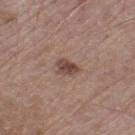No biopsy was performed on this lesion — it was imaged during a full skin examination and was not determined to be concerning.
A lesion tile, about 15 mm wide, cut from a 3D total-body photograph.
The lesion is on the left thigh.
The patient is a male about 65 years old.